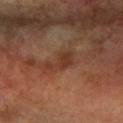Impression:
Part of a total-body skin-imaging series; this lesion was reviewed on a skin check and was not flagged for biopsy.
Acquisition and patient details:
The lesion's longest dimension is about 4 mm. A 15 mm crop from a total-body photograph taken for skin-cancer surveillance. The lesion is located on the left forearm. A female patient, approximately 60 years of age. This is a cross-polarized tile.